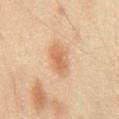Assessment: This lesion was catalogued during total-body skin photography and was not selected for biopsy. Background: An algorithmic analysis of the crop reported a mean CIELAB color near L≈52 a*≈17 b*≈31 and a normalized border contrast of about 6.5. The analysis additionally found an automated nevus-likeness rating near 5 out of 100. Cropped from a total-body skin-imaging series; the visible field is about 15 mm. The lesion's longest dimension is about 4.5 mm. The lesion is on the abdomen. The subject is a male aged 43 to 47.